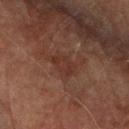follow-up = no biopsy performed (imaged during a skin exam)
anatomic site = the right upper arm
automated metrics = an area of roughly 4.5 mm², an eccentricity of roughly 0.8, and two-axis asymmetry of about 0.3; a normalized lesion–skin contrast near 5.5; a detector confidence of about 100 out of 100 that the crop contains a lesion
acquisition = 15 mm crop, total-body photography
tile lighting = cross-polarized illumination
patient = male, in their mid- to late 70s
lesion size = ~3 mm (longest diameter)